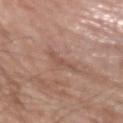Part of a total-body skin-imaging series; this lesion was reviewed on a skin check and was not flagged for biopsy. The lesion is on the right upper arm. A male patient aged approximately 75. Measured at roughly 3 mm in maximum diameter. The tile uses white-light illumination. Cropped from a total-body skin-imaging series; the visible field is about 15 mm.